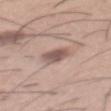workup: imaged on a skin check; not biopsied.